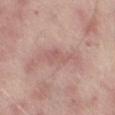Imaged during a routine full-body skin examination; the lesion was not biopsied and no histopathology is available. From the left thigh. A 15 mm close-up tile from a total-body photography series done for melanoma screening. A male patient approximately 65 years of age. The tile uses white-light illumination. The recorded lesion diameter is about 3.5 mm.